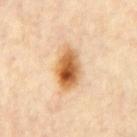Imaged during a routine full-body skin examination; the lesion was not biopsied and no histopathology is available. An algorithmic analysis of the crop reported a lesion area of about 13 mm², an outline eccentricity of about 0.8 (0 = round, 1 = elongated), and two-axis asymmetry of about 0.2. And it measured about 16 CIELAB-L* units darker than the surrounding skin. The software also gave border irregularity of about 2 on a 0–10 scale and radial color variation of about 3. Imaged with cross-polarized lighting. The lesion is located on the chest. A male subject about 60 years old. Longest diameter approximately 5 mm. Cropped from a whole-body photographic skin survey; the tile spans about 15 mm.